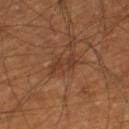notes — total-body-photography surveillance lesion; no biopsy
lesion size — ≈3 mm
imaging modality — ~15 mm crop, total-body skin-cancer survey
subject — male, aged around 60
illumination — cross-polarized
anatomic site — the right lower leg